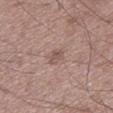biopsy status: imaged on a skin check; not biopsied
lighting: white-light illumination
anatomic site: the left lower leg
subject: male, about 50 years old
image-analysis metrics: an area of roughly 4 mm², a shape eccentricity near 0.75, and a shape-asymmetry score of about 0.25 (0 = symmetric); an automated nevus-likeness rating near 0 out of 100 and a lesion-detection confidence of about 100/100
imaging modality: ~15 mm crop, total-body skin-cancer survey
lesion diameter: ≈2.5 mm